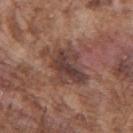Recorded during total-body skin imaging; not selected for excision or biopsy.
A 15 mm crop from a total-body photograph taken for skin-cancer surveillance.
A male patient, aged around 75.
On the arm.
Automated tile analysis of the lesion measured a border-irregularity rating of about 4.5/10, internal color variation of about 5.5 on a 0–10 scale, and a peripheral color-asymmetry measure near 2.
This is a white-light tile.
Approximately 5.5 mm at its widest.